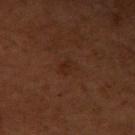biopsy status: catalogued during a skin exam; not biopsied | imaging modality: 15 mm crop, total-body photography | site: the right upper arm | TBP lesion metrics: border irregularity of about 2 on a 0–10 scale and a within-lesion color-variation index near 1.5/10 | patient: male, about 60 years old | lesion size: ~2.5 mm (longest diameter) | tile lighting: cross-polarized.